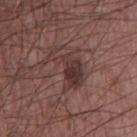notes: total-body-photography surveillance lesion; no biopsy | site: the chest | acquisition: total-body-photography crop, ~15 mm field of view | image-analysis metrics: an average lesion color of about L≈35 a*≈19 b*≈20 (CIELAB) and about 8 CIELAB-L* units darker than the surrounding skin; a border-irregularity index near 8.5/10, a color-variation rating of about 4.5/10, and peripheral color asymmetry of about 1.5 | patient: male, aged 58 to 62 | lighting: white-light illumination | lesion size: ~5.5 mm (longest diameter).